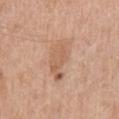workup — total-body-photography surveillance lesion; no biopsy | diameter — ~5.5 mm (longest diameter) | illumination — white-light illumination | acquisition — total-body-photography crop, ~15 mm field of view | subject — male, aged around 65 | location — the front of the torso.